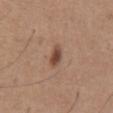| field | value |
|---|---|
| anatomic site | the abdomen |
| patient | male, aged around 65 |
| image | ~15 mm tile from a whole-body skin photo |
| lesion size | ~3 mm (longest diameter) |
| automated lesion analysis | a mean CIELAB color near L≈47 a*≈20 b*≈28, about 12 CIELAB-L* units darker than the surrounding skin, and a lesion-to-skin contrast of about 8.5 (normalized; higher = more distinct) |